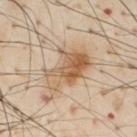Part of a total-body skin-imaging series; this lesion was reviewed on a skin check and was not flagged for biopsy. Cropped from a total-body skin-imaging series; the visible field is about 15 mm. The subject is a male aged approximately 55. On the chest.